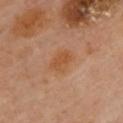workup: total-body-photography surveillance lesion; no biopsy
lesion diameter: about 3.5 mm
location: the chest
illumination: cross-polarized illumination
subject: female, aged 58 to 62
imaging modality: total-body-photography crop, ~15 mm field of view